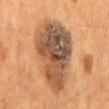Clinical summary:
A male patient, approximately 55 years of age. A roughly 15 mm field-of-view crop from a total-body skin photograph. Longest diameter approximately 9 mm. Located on the mid back.